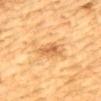Part of a total-body skin-imaging series; this lesion was reviewed on a skin check and was not flagged for biopsy. The lesion is on the mid back. A close-up tile cropped from a whole-body skin photograph, about 15 mm across. A male patient about 85 years old or older.